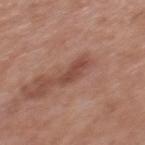Clinical impression:
Captured during whole-body skin photography for melanoma surveillance; the lesion was not biopsied.
Image and clinical context:
Located on the mid back. A male subject approximately 70 years of age. The lesion's longest dimension is about 3 mm. Imaged with white-light lighting. A 15 mm crop from a total-body photograph taken for skin-cancer surveillance.